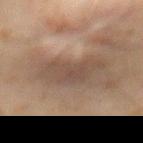follow-up — imaged on a skin check; not biopsied
anatomic site — the abdomen
subject — male, aged around 60
lesion size — ~6 mm (longest diameter)
image — total-body-photography crop, ~15 mm field of view
image-analysis metrics — an area of roughly 15 mm² and two-axis asymmetry of about 0.35; an average lesion color of about L≈39 a*≈13 b*≈21 (CIELAB); a classifier nevus-likeness of about 0/100
lighting — cross-polarized illumination